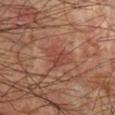Clinical summary:
A male subject aged around 75. From the left upper arm. The lesion's longest dimension is about 3 mm. Automated image analysis of the tile measured an area of roughly 6.5 mm² and two-axis asymmetry of about 0.35. The software also gave a detector confidence of about 100 out of 100 that the crop contains a lesion. Captured under cross-polarized illumination. A region of skin cropped from a whole-body photographic capture, roughly 15 mm wide.